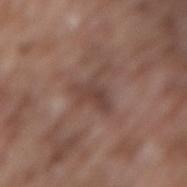The lesion was photographed on a routine skin check and not biopsied; there is no pathology result. A lesion tile, about 15 mm wide, cut from a 3D total-body photograph. A male subject, in their mid- to late 70s. The lesion is located on the back.